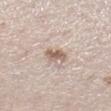{
  "biopsy_status": "not biopsied; imaged during a skin examination",
  "lighting": "white-light",
  "patient": {
    "sex": "female",
    "age_approx": 40
  },
  "lesion_size": {
    "long_diameter_mm_approx": 2.5
  },
  "image": {
    "source": "total-body photography crop",
    "field_of_view_mm": 15
  },
  "automated_metrics": {
    "border_irregularity_0_10": 3.0,
    "color_variation_0_10": 1.5,
    "peripheral_color_asymmetry": 0.5,
    "nevus_likeness_0_100": 35,
    "lesion_detection_confidence_0_100": 95
  },
  "site": "left lower leg"
}